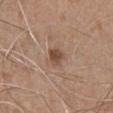Q: Was this lesion biopsied?
A: total-body-photography surveillance lesion; no biopsy
Q: What kind of image is this?
A: total-body-photography crop, ~15 mm field of view
Q: What is the anatomic site?
A: the chest
Q: Who is the patient?
A: male, about 55 years old